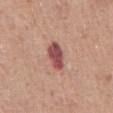Assessment:
Captured during whole-body skin photography for melanoma surveillance; the lesion was not biopsied.
Context:
Automated tile analysis of the lesion measured a lesion area of about 7 mm² and a shape eccentricity near 0.8. The analysis additionally found a nevus-likeness score of about 35/100 and a detector confidence of about 100 out of 100 that the crop contains a lesion. The lesion is on the abdomen. A male patient, approximately 50 years of age. Imaged with white-light lighting. Cropped from a total-body skin-imaging series; the visible field is about 15 mm. The recorded lesion diameter is about 3.5 mm.